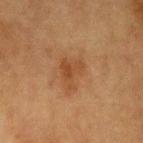<case>
  <biopsy_status>not biopsied; imaged during a skin examination</biopsy_status>
  <lesion_size>
    <long_diameter_mm_approx>3.5</long_diameter_mm_approx>
  </lesion_size>
  <image>
    <source>total-body photography crop</source>
    <field_of_view_mm>15</field_of_view_mm>
  </image>
  <site>left upper arm</site>
  <lighting>cross-polarized</lighting>
  <patient>
    <sex>female</sex>
    <age_approx>55</age_approx>
  </patient>
</case>